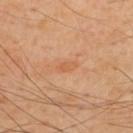Q: Was a biopsy performed?
A: catalogued during a skin exam; not biopsied
Q: What did automated image analysis measure?
A: a lesion area of about 2.5 mm² and a symmetry-axis asymmetry near 0.2; a lesion–skin lightness drop of about 6 and a lesion-to-skin contrast of about 5 (normalized; higher = more distinct)
Q: What is the lesion's diameter?
A: ~2.5 mm (longest diameter)
Q: Illumination type?
A: cross-polarized
Q: What is the anatomic site?
A: the back
Q: How was this image acquired?
A: ~15 mm crop, total-body skin-cancer survey
Q: What are the patient's age and sex?
A: male, in their 50s